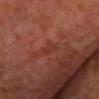Part of a total-body skin-imaging series; this lesion was reviewed on a skin check and was not flagged for biopsy.
Automated image analysis of the tile measured an area of roughly 2.5 mm² and a symmetry-axis asymmetry near 0.4.
Captured under cross-polarized illumination.
The lesion's longest dimension is about 2.5 mm.
A roughly 15 mm field-of-view crop from a total-body skin photograph.
A male subject, in their mid-60s.
The lesion is located on the chest.